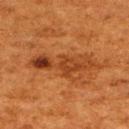Impression:
Part of a total-body skin-imaging series; this lesion was reviewed on a skin check and was not flagged for biopsy.
Acquisition and patient details:
The lesion-visualizer software estimated an area of roughly 15 mm² and two-axis asymmetry of about 0.45. And it measured a border-irregularity rating of about 8/10, a within-lesion color-variation index near 7.5/10, and peripheral color asymmetry of about 2.5. The analysis additionally found a nevus-likeness score of about 15/100 and lesion-presence confidence of about 100/100. The recorded lesion diameter is about 8.5 mm. A region of skin cropped from a whole-body photographic capture, roughly 15 mm wide. The patient is a female roughly 50 years of age. Located on the back.